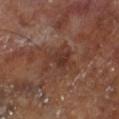Captured during whole-body skin photography for melanoma surveillance; the lesion was not biopsied.
From the right lower leg.
A male patient about 70 years old.
Cropped from a total-body skin-imaging series; the visible field is about 15 mm.
Automated tile analysis of the lesion measured a lesion area of about 11 mm², a shape eccentricity near 0.5, and a symmetry-axis asymmetry near 0.45. The analysis additionally found an average lesion color of about L≈33 a*≈19 b*≈24 (CIELAB), a lesion–skin lightness drop of about 7, and a lesion-to-skin contrast of about 6.5 (normalized; higher = more distinct).
This is a cross-polarized tile.
Measured at roughly 4.5 mm in maximum diameter.